On the abdomen. This is a white-light tile. This image is a 15 mm lesion crop taken from a total-body photograph. A male subject, aged approximately 65. Approximately 6.5 mm at its widest: 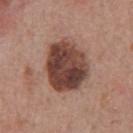  diagnosis:
    histopathology: atypical intraepithelial melanocytic proliferation
    malignancy: indeterminate
    taxonomic_path:
      - Indeterminate
      - Indeterminate melanocytic proliferations
      - Atypical intraepithelial melanocytic proliferation